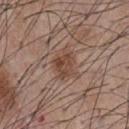workup: no biopsy performed (imaged during a skin exam); automated lesion analysis: a footprint of about 7.5 mm², an outline eccentricity of about 0.7 (0 = round, 1 = elongated), and two-axis asymmetry of about 0.25; diameter: ≈3.5 mm; image source: total-body-photography crop, ~15 mm field of view; patient: male, aged around 55; anatomic site: the chest; illumination: white-light.